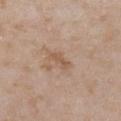No biopsy was performed on this lesion — it was imaged during a full skin examination and was not determined to be concerning. The total-body-photography lesion software estimated an average lesion color of about L≈55 a*≈17 b*≈31 (CIELAB) and a normalized lesion–skin contrast near 6. And it measured border irregularity of about 3 on a 0–10 scale and a within-lesion color-variation index near 0/10. The lesion is on the chest. A 15 mm close-up extracted from a 3D total-body photography capture. The recorded lesion diameter is about 2.5 mm. The patient is a female aged around 30. This is a white-light tile.